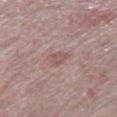Clinical summary: On the right thigh. Cropped from a total-body skin-imaging series; the visible field is about 15 mm. The patient is a male in their mid- to late 70s.